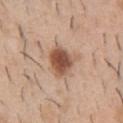anatomic site: the chest | subject: male, aged 38 to 42 | acquisition: total-body-photography crop, ~15 mm field of view.